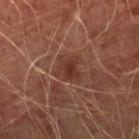Clinical impression: Captured during whole-body skin photography for melanoma surveillance; the lesion was not biopsied. Background: About 3 mm across. A male patient, aged approximately 75. Imaged with cross-polarized lighting. The lesion is on the right lower leg. A close-up tile cropped from a whole-body skin photograph, about 15 mm across.